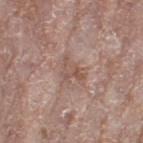Clinical impression:
Captured during whole-body skin photography for melanoma surveillance; the lesion was not biopsied.
Background:
This is a white-light tile. A close-up tile cropped from a whole-body skin photograph, about 15 mm across. An algorithmic analysis of the crop reported a footprint of about 5.5 mm², an outline eccentricity of about 0.75 (0 = round, 1 = elongated), and a symmetry-axis asymmetry near 0.5. The analysis additionally found a border-irregularity rating of about 6.5/10, internal color variation of about 2.5 on a 0–10 scale, and a peripheral color-asymmetry measure near 1. Measured at roughly 4 mm in maximum diameter. On the left thigh. The subject is a female roughly 75 years of age.